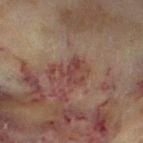Q: Was this lesion biopsied?
A: imaged on a skin check; not biopsied
Q: Lesion location?
A: the left thigh
Q: What is the imaging modality?
A: 15 mm crop, total-body photography
Q: What are the patient's age and sex?
A: female, about 60 years old